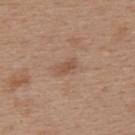  biopsy_status: not biopsied; imaged during a skin examination
  image:
    source: total-body photography crop
    field_of_view_mm: 15
  lighting: white-light
  site: upper back
  patient:
    sex: female
    age_approx: 40